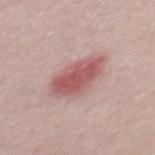Impression: Captured during whole-body skin photography for melanoma surveillance; the lesion was not biopsied. Clinical summary: The patient is a male aged around 30. Approximately 5.5 mm at its widest. The total-body-photography lesion software estimated a border-irregularity rating of about 2.5/10. The software also gave a classifier nevus-likeness of about 35/100. The tile uses white-light illumination. This image is a 15 mm lesion crop taken from a total-body photograph.